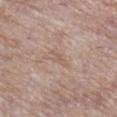<case>
<biopsy_status>not biopsied; imaged during a skin examination</biopsy_status>
<lighting>white-light</lighting>
<patient>
  <sex>female</sex>
  <age_approx>70</age_approx>
</patient>
<site>right lower leg</site>
<lesion_size>
  <long_diameter_mm_approx>2.5</long_diameter_mm_approx>
</lesion_size>
<image>
  <source>total-body photography crop</source>
  <field_of_view_mm>15</field_of_view_mm>
</image>
</case>